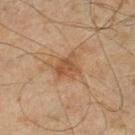Assessment: Recorded during total-body skin imaging; not selected for excision or biopsy. Context: The lesion is on the leg. A close-up tile cropped from a whole-body skin photograph, about 15 mm across. A male patient, approximately 45 years of age.